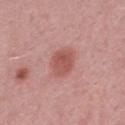biopsy status = imaged on a skin check; not biopsied
illumination = white-light
location = the mid back
imaging modality = total-body-photography crop, ~15 mm field of view
size = about 3.5 mm
patient = male, aged 48–52